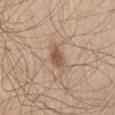The lesion was tiled from a total-body skin photograph and was not biopsied. A 15 mm close-up extracted from a 3D total-body photography capture. A male subject, in their 60s. Located on the right thigh.